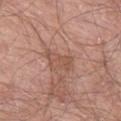Recorded during total-body skin imaging; not selected for excision or biopsy. A 15 mm close-up tile from a total-body photography series done for melanoma screening. Automated image analysis of the tile measured a lesion area of about 5 mm² and two-axis asymmetry of about 0.4. The software also gave an average lesion color of about L≈53 a*≈21 b*≈28 (CIELAB), about 7 CIELAB-L* units darker than the surrounding skin, and a normalized border contrast of about 5.5. Longest diameter approximately 3.5 mm. The lesion is on the left thigh. The tile uses white-light illumination. The patient is a male aged approximately 70.